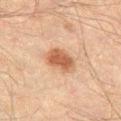Recorded during total-body skin imaging; not selected for excision or biopsy.
This image is a 15 mm lesion crop taken from a total-body photograph.
The total-body-photography lesion software estimated a mean CIELAB color near L≈45 a*≈19 b*≈29, roughly 11 lightness units darker than nearby skin, and a lesion-to-skin contrast of about 9 (normalized; higher = more distinct).
From the left thigh.
The patient is a male roughly 60 years of age.
Imaged with cross-polarized lighting.
The recorded lesion diameter is about 4 mm.